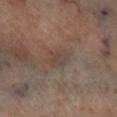Assessment:
The lesion was photographed on a routine skin check and not biopsied; there is no pathology result.
Clinical summary:
An algorithmic analysis of the crop reported a footprint of about 5 mm², an eccentricity of roughly 0.75, and a symmetry-axis asymmetry near 0.25. This is a cross-polarized tile. Cropped from a total-body skin-imaging series; the visible field is about 15 mm. Located on the leg. About 3 mm across.